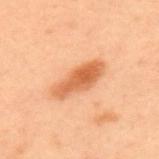Recorded during total-body skin imaging; not selected for excision or biopsy. This image is a 15 mm lesion crop taken from a total-body photograph. From the upper back. A male subject in their mid-50s.